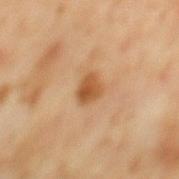Findings:
- follow-up · catalogued during a skin exam; not biopsied
- lesion diameter · ≈2.5 mm
- anatomic site · the mid back
- acquisition · ~15 mm crop, total-body skin-cancer survey
- subject · male, about 60 years old
- illumination · cross-polarized illumination
- TBP lesion metrics · a normalized border contrast of about 8.5; an automated nevus-likeness rating near 85 out of 100 and a lesion-detection confidence of about 100/100